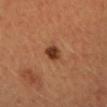follow-up — no biopsy performed (imaged during a skin exam) | patient — male, aged around 20 | diameter — ≈2.5 mm | image source — 15 mm crop, total-body photography | illumination — cross-polarized | location — the head or neck.